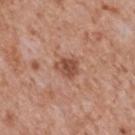<record>
  <biopsy_status>not biopsied; imaged during a skin examination</biopsy_status>
  <image>
    <source>total-body photography crop</source>
    <field_of_view_mm>15</field_of_view_mm>
  </image>
  <site>mid back</site>
  <patient>
    <sex>male</sex>
    <age_approx>65</age_approx>
  </patient>
  <lesion_size>
    <long_diameter_mm_approx>2.5</long_diameter_mm_approx>
  </lesion_size>
</record>